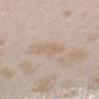The lesion was photographed on a routine skin check and not biopsied; there is no pathology result.
The lesion's longest dimension is about 3.5 mm.
A female patient in their mid- to late 20s.
From the arm.
Imaged with white-light lighting.
This image is a 15 mm lesion crop taken from a total-body photograph.
Automated image analysis of the tile measured a lesion area of about 4.5 mm², an eccentricity of roughly 0.9, and a shape-asymmetry score of about 0.25 (0 = symmetric). The analysis additionally found an average lesion color of about L≈64 a*≈13 b*≈28 (CIELAB) and about 6 CIELAB-L* units darker than the surrounding skin.